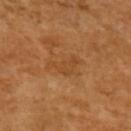Captured under cross-polarized illumination. The recorded lesion diameter is about 4 mm. The patient is a female approximately 55 years of age. A 15 mm close-up extracted from a 3D total-body photography capture. The lesion is located on the arm.